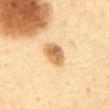Q: Is there a histopathology result?
A: imaged on a skin check; not biopsied
Q: What are the patient's age and sex?
A: male, aged around 60
Q: How was this image acquired?
A: ~15 mm tile from a whole-body skin photo
Q: What lighting was used for the tile?
A: cross-polarized illumination
Q: Where on the body is the lesion?
A: the abdomen